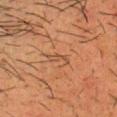Recorded during total-body skin imaging; not selected for excision or biopsy.
On the head or neck.
Longest diameter approximately 3 mm.
A 15 mm crop from a total-body photograph taken for skin-cancer surveillance.
A male patient in their mid-30s.
The lesion-visualizer software estimated a lesion area of about 3 mm² and a shape eccentricity near 0.95. The analysis additionally found a mean CIELAB color near L≈39 a*≈18 b*≈28, about 6 CIELAB-L* units darker than the surrounding skin, and a normalized lesion–skin contrast near 5.